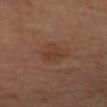biopsy_status: not biopsied; imaged during a skin examination
lesion_size:
  long_diameter_mm_approx: 3.0
image:
  source: total-body photography crop
  field_of_view_mm: 15
patient:
  sex: female
  age_approx: 65
lighting: cross-polarized
automated_metrics:
  area_mm2_approx: 6.0
  eccentricity: 0.5
  shape_asymmetry: 0.25
  cielab_L: 37
  cielab_a: 20
  cielab_b: 27
  vs_skin_darker_L: 5.0
  vs_skin_contrast_norm: 5.0
  border_irregularity_0_10: 2.5
  color_variation_0_10: 2.0
  peripheral_color_asymmetry: 1.0
site: left forearm